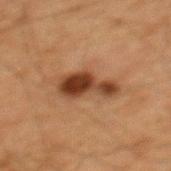Recorded during total-body skin imaging; not selected for excision or biopsy. A male subject aged around 65. The recorded lesion diameter is about 5.5 mm. The lesion is on the mid back. Cropped from a total-body skin-imaging series; the visible field is about 15 mm.